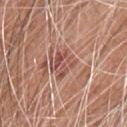biopsy status: imaged on a skin check; not biopsied | diameter: ~3.5 mm (longest diameter) | body site: the chest | acquisition: ~15 mm crop, total-body skin-cancer survey | subject: male, aged approximately 60.